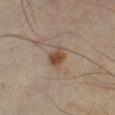A male subject in their 40s.
Approximately 2.5 mm at its widest.
Captured under cross-polarized illumination.
A 15 mm crop from a total-body photograph taken for skin-cancer surveillance.
From the right lower leg.